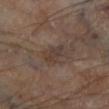The lesion was photographed on a routine skin check and not biopsied; there is no pathology result. The lesion is located on the left lower leg. The tile uses cross-polarized illumination. A roughly 15 mm field-of-view crop from a total-body skin photograph. A male subject, aged around 70.